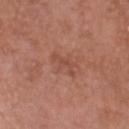Impression: No biopsy was performed on this lesion — it was imaged during a full skin examination and was not determined to be concerning. Acquisition and patient details: The lesion is located on the head or neck. A male subject, aged 48–52. The total-body-photography lesion software estimated a lesion area of about 3.5 mm², an eccentricity of roughly 0.9, and a symmetry-axis asymmetry near 0.5. The recorded lesion diameter is about 3 mm. A 15 mm close-up tile from a total-body photography series done for melanoma screening.